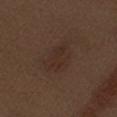Assessment:
The lesion was photographed on a routine skin check and not biopsied; there is no pathology result.
Image and clinical context:
Longest diameter approximately 4.5 mm. A male subject aged approximately 70. Imaged with white-light lighting. Cropped from a whole-body photographic skin survey; the tile spans about 15 mm. On the left upper arm.